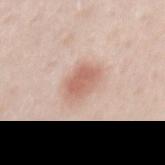Imaged during a routine full-body skin examination; the lesion was not biopsied and no histopathology is available.
A male subject, in their 40s.
This is a white-light tile.
Located on the mid back.
About 4 mm across.
This image is a 15 mm lesion crop taken from a total-body photograph.